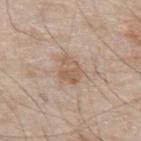Findings:
* notes: catalogued during a skin exam; not biopsied
* size: ~3 mm (longest diameter)
* body site: the abdomen
* patient: male, approximately 80 years of age
* image source: ~15 mm crop, total-body skin-cancer survey
* tile lighting: white-light illumination